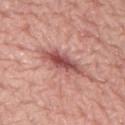The total-body-photography lesion software estimated an area of roughly 10 mm² and an eccentricity of roughly 0.95. And it measured a lesion color around L≈53 a*≈27 b*≈24 in CIELAB, about 13 CIELAB-L* units darker than the surrounding skin, and a normalized lesion–skin contrast near 8.5. It also reported a nevus-likeness score of about 10/100 and a detector confidence of about 75 out of 100 that the crop contains a lesion.
The patient is a male in their 70s.
This is a white-light tile.
Located on the left lower leg.
Cropped from a whole-body photographic skin survey; the tile spans about 15 mm.
Measured at roughly 6 mm in maximum diameter.